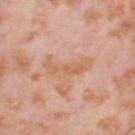Impression: Recorded during total-body skin imaging; not selected for excision or biopsy. Clinical summary: A female subject, approximately 55 years of age. This is a cross-polarized tile. Cropped from a whole-body photographic skin survey; the tile spans about 15 mm. Approximately 4.5 mm at its widest. Located on the right thigh.